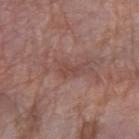Part of a total-body skin-imaging series; this lesion was reviewed on a skin check and was not flagged for biopsy.
A female patient, in their 70s.
A 15 mm close-up tile from a total-body photography series done for melanoma screening.
Imaged with white-light lighting.
About 3 mm across.
An algorithmic analysis of the crop reported a shape eccentricity near 0.9 and two-axis asymmetry of about 0.4. The software also gave a mean CIELAB color near L≈45 a*≈21 b*≈24 and a lesion-to-skin contrast of about 5.5 (normalized; higher = more distinct). It also reported a border-irregularity rating of about 5/10, a within-lesion color-variation index near 0/10, and radial color variation of about 0.
On the right forearm.